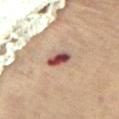This lesion was catalogued during total-body skin photography and was not selected for biopsy. The recorded lesion diameter is about 3.5 mm. Automated tile analysis of the lesion measured a lesion area of about 5 mm², a shape eccentricity near 0.85, and a symmetry-axis asymmetry near 0.2. And it measured a mean CIELAB color near L≈43 a*≈21 b*≈22, about 16 CIELAB-L* units darker than the surrounding skin, and a normalized border contrast of about 12.5. The software also gave border irregularity of about 2 on a 0–10 scale, a within-lesion color-variation index near 6.5/10, and a peripheral color-asymmetry measure near 2. It also reported an automated nevus-likeness rating near 5 out of 100. The lesion is on the front of the torso. Imaged with cross-polarized lighting. The subject is a female aged 68 to 72. A region of skin cropped from a whole-body photographic capture, roughly 15 mm wide.